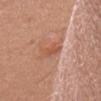workup — catalogued during a skin exam; not biopsied | tile lighting — white-light | location — the head or neck | diameter — ~2.5 mm (longest diameter) | patient — female, approximately 50 years of age | TBP lesion metrics — a lesion area of about 5 mm², a shape eccentricity near 0.7, and two-axis asymmetry of about 0.2; an average lesion color of about L≈55 a*≈25 b*≈33 (CIELAB), roughly 8 lightness units darker than nearby skin, and a normalized border contrast of about 6; a nevus-likeness score of about 60/100 | image source — ~15 mm tile from a whole-body skin photo.